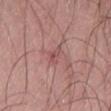Notes:
- follow-up — imaged on a skin check; not biopsied
- patient — male, aged 48 to 52
- location — the lower back
- image source — ~15 mm crop, total-body skin-cancer survey
- diameter — ≈2.5 mm
- image-analysis metrics — a footprint of about 2.5 mm², an eccentricity of roughly 0.9, and two-axis asymmetry of about 0.5; a lesion color around L≈51 a*≈25 b*≈21 in CIELAB, roughly 8 lightness units darker than nearby skin, and a normalized lesion–skin contrast near 5.5; border irregularity of about 5 on a 0–10 scale and a within-lesion color-variation index near 1/10; a classifier nevus-likeness of about 0/100 and lesion-presence confidence of about 85/100
- lighting — white-light illumination